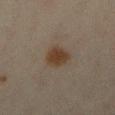Clinical impression:
Captured during whole-body skin photography for melanoma surveillance; the lesion was not biopsied.
Acquisition and patient details:
A female patient aged around 45. Measured at roughly 3.5 mm in maximum diameter. On the right lower leg. Cropped from a total-body skin-imaging series; the visible field is about 15 mm. The tile uses cross-polarized illumination.